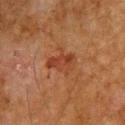Captured during whole-body skin photography for melanoma surveillance; the lesion was not biopsied.
The total-body-photography lesion software estimated a footprint of about 7 mm² and an eccentricity of roughly 0.75. The software also gave a lesion color around L≈33 a*≈23 b*≈29 in CIELAB, roughly 7 lightness units darker than nearby skin, and a lesion-to-skin contrast of about 6.5 (normalized; higher = more distinct).
On the upper back.
This image is a 15 mm lesion crop taken from a total-body photograph.
Measured at roughly 3.5 mm in maximum diameter.
A male patient, approximately 65 years of age.
Imaged with cross-polarized lighting.